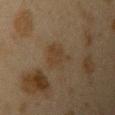Assessment:
The lesion was tiled from a total-body skin photograph and was not biopsied.
Clinical summary:
A roughly 15 mm field-of-view crop from a total-body skin photograph. A female patient, approximately 40 years of age. Located on the left upper arm. An algorithmic analysis of the crop reported a footprint of about 8.5 mm², an outline eccentricity of about 0.55 (0 = round, 1 = elongated), and two-axis asymmetry of about 0.3. The software also gave an average lesion color of about L≈31 a*≈11 b*≈25 (CIELAB), roughly 4 lightness units darker than nearby skin, and a lesion-to-skin contrast of about 5.5 (normalized; higher = more distinct). It also reported a border-irregularity index near 3.5/10, internal color variation of about 2 on a 0–10 scale, and a peripheral color-asymmetry measure near 0.5. Imaged with cross-polarized lighting. Longest diameter approximately 3.5 mm.